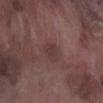• biopsy status · catalogued during a skin exam; not biopsied
• patient · male, aged 73 to 77
• anatomic site · the left lower leg
• image source · ~15 mm tile from a whole-body skin photo